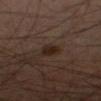Impression: Recorded during total-body skin imaging; not selected for excision or biopsy. Image and clinical context: The lesion is located on the right forearm. A male subject about 45 years old. A roughly 15 mm field-of-view crop from a total-body skin photograph.